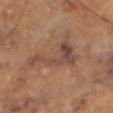No biopsy was performed on this lesion — it was imaged during a full skin examination and was not determined to be concerning.
The recorded lesion diameter is about 6.5 mm.
From the right thigh.
A male patient, aged around 75.
A close-up tile cropped from a whole-body skin photograph, about 15 mm across.